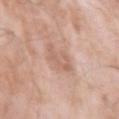notes: imaged on a skin check; not biopsied | image: ~15 mm crop, total-body skin-cancer survey | site: the left upper arm | subject: male, aged around 75.